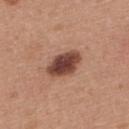workup = no biopsy performed (imaged during a skin exam) | size = about 4.5 mm | tile lighting = white-light illumination | acquisition = 15 mm crop, total-body photography | patient = female, aged 33–37 | anatomic site = the upper back | TBP lesion metrics = a mean CIELAB color near L≈44 a*≈23 b*≈26 and a lesion–skin lightness drop of about 17; an automated nevus-likeness rating near 95 out of 100.